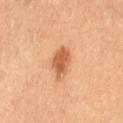follow-up=catalogued during a skin exam; not biopsied
automated lesion analysis=a footprint of about 7 mm², a shape eccentricity near 0.7, and a symmetry-axis asymmetry near 0.25; border irregularity of about 2.5 on a 0–10 scale and a color-variation rating of about 2.5/10
lighting=cross-polarized
patient=female, roughly 60 years of age
site=the left thigh
image=~15 mm crop, total-body skin-cancer survey